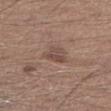Recorded during total-body skin imaging; not selected for excision or biopsy.
Longest diameter approximately 3 mm.
The lesion is on the left lower leg.
The total-body-photography lesion software estimated a lesion area of about 5 mm², a shape eccentricity near 0.6, and two-axis asymmetry of about 0.35. And it measured a mean CIELAB color near L≈47 a*≈17 b*≈23, about 8 CIELAB-L* units darker than the surrounding skin, and a normalized border contrast of about 6.5. It also reported a border-irregularity rating of about 3.5/10 and peripheral color asymmetry of about 1. And it measured an automated nevus-likeness rating near 5 out of 100 and a lesion-detection confidence of about 100/100.
This is a white-light tile.
A 15 mm close-up extracted from a 3D total-body photography capture.
The patient is a male in their 30s.